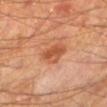About 3 mm across.
Captured under cross-polarized illumination.
The subject is a male approximately 65 years of age.
A lesion tile, about 15 mm wide, cut from a 3D total-body photograph.
Automated tile analysis of the lesion measured a footprint of about 7.5 mm², an outline eccentricity of about 0.5 (0 = round, 1 = elongated), and two-axis asymmetry of about 0.3. The analysis additionally found an automated nevus-likeness rating near 70 out of 100 and a detector confidence of about 100 out of 100 that the crop contains a lesion.
From the left lower leg.